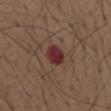| field | value |
|---|---|
| workup | imaged on a skin check; not biopsied |
| tile lighting | white-light |
| image source | ~15 mm tile from a whole-body skin photo |
| site | the abdomen |
| patient | male, aged 73–77 |
| lesion size | ≈2.5 mm |